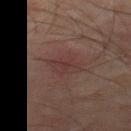notes: catalogued during a skin exam; not biopsied | lesion size: about 3.5 mm | image source: 15 mm crop, total-body photography | patient: male, aged around 75 | location: the leg | tile lighting: cross-polarized illumination | automated metrics: an area of roughly 7 mm², an outline eccentricity of about 0.75 (0 = round, 1 = elongated), and a symmetry-axis asymmetry near 0.3; an automated nevus-likeness rating near 5 out of 100.